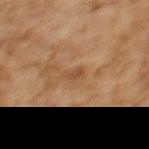Clinical impression:
Captured during whole-body skin photography for melanoma surveillance; the lesion was not biopsied.
Background:
From the upper back. The recorded lesion diameter is about 2.5 mm. This image is a 15 mm lesion crop taken from a total-body photograph. Imaged with cross-polarized lighting. A female subject aged around 60. Automated image analysis of the tile measured a lesion area of about 2 mm². The software also gave an average lesion color of about L≈42 a*≈18 b*≈31 (CIELAB), roughly 7 lightness units darker than nearby skin, and a normalized border contrast of about 6.